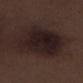patient=male, roughly 70 years of age
acquisition=15 mm crop, total-body photography
size=≈8 mm
lighting=white-light illumination
site=the right lower leg